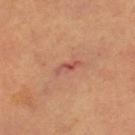Q: Was this lesion biopsied?
A: total-body-photography surveillance lesion; no biopsy
Q: Automated lesion metrics?
A: a border-irregularity index near 4/10
Q: What is the lesion's diameter?
A: ~3 mm (longest diameter)
Q: What lighting was used for the tile?
A: cross-polarized
Q: What kind of image is this?
A: ~15 mm tile from a whole-body skin photo
Q: What is the anatomic site?
A: the right leg
Q: Who is the patient?
A: female, aged 43–47